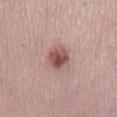Q: What kind of image is this?
A: total-body-photography crop, ~15 mm field of view
Q: Lesion location?
A: the right lower leg
Q: What are the patient's age and sex?
A: female, approximately 40 years of age